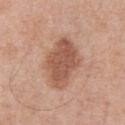The lesion was tiled from a total-body skin photograph and was not biopsied.
Located on the front of the torso.
A 15 mm close-up extracted from a 3D total-body photography capture.
This is a white-light tile.
The subject is a male approximately 70 years of age.
About 6 mm across.
Automated tile analysis of the lesion measured a lesion color around L≈54 a*≈22 b*≈30 in CIELAB, about 12 CIELAB-L* units darker than the surrounding skin, and a lesion-to-skin contrast of about 8 (normalized; higher = more distinct). It also reported a detector confidence of about 100 out of 100 that the crop contains a lesion.